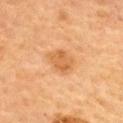The lesion was tiled from a total-body skin photograph and was not biopsied. A female patient, aged around 60. From the upper back. A 15 mm close-up tile from a total-body photography series done for melanoma screening.